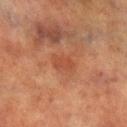Notes:
– notes · imaged on a skin check; not biopsied
– imaging modality · 15 mm crop, total-body photography
– lesion size · about 3 mm
– location · the left lower leg
– tile lighting · cross-polarized
– subject · male, approximately 70 years of age
– TBP lesion metrics · border irregularity of about 3 on a 0–10 scale, a within-lesion color-variation index near 1.5/10, and peripheral color asymmetry of about 0.5; a classifier nevus-likeness of about 0/100 and a lesion-detection confidence of about 100/100